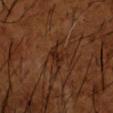Q: Was this lesion biopsied?
A: no biopsy performed (imaged during a skin exam)
Q: Patient demographics?
A: male, aged 63 to 67
Q: What kind of image is this?
A: ~15 mm crop, total-body skin-cancer survey
Q: Automated lesion metrics?
A: a footprint of about 3.5 mm², a shape eccentricity near 0.75, and a shape-asymmetry score of about 0.3 (0 = symmetric); a nevus-likeness score of about 0/100 and a lesion-detection confidence of about 95/100
Q: Lesion location?
A: the arm